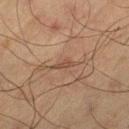Imaged during a routine full-body skin examination; the lesion was not biopsied and no histopathology is available.
About 2.5 mm across.
A male subject in their mid-60s.
From the leg.
Automated tile analysis of the lesion measured a lesion area of about 2 mm², an outline eccentricity of about 0.9 (0 = round, 1 = elongated), and a symmetry-axis asymmetry near 0.35. The software also gave a classifier nevus-likeness of about 0/100.
A lesion tile, about 15 mm wide, cut from a 3D total-body photograph.
The tile uses cross-polarized illumination.